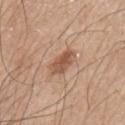This lesion was catalogued during total-body skin photography and was not selected for biopsy. The lesion-visualizer software estimated a lesion area of about 6 mm² and a shape eccentricity near 0.8. The software also gave a mean CIELAB color near L≈54 a*≈20 b*≈31, a lesion–skin lightness drop of about 11, and a normalized border contrast of about 8. The lesion is located on the left upper arm. The tile uses white-light illumination. Cropped from a total-body skin-imaging series; the visible field is about 15 mm. The patient is a male roughly 75 years of age.